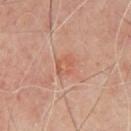Findings:
- notes · total-body-photography surveillance lesion; no biopsy
- tile lighting · cross-polarized illumination
- image source · total-body-photography crop, ~15 mm field of view
- subject · male, aged 63 to 67
- lesion size · ≈2.5 mm
- location · the front of the torso